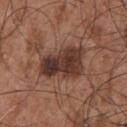The lesion was photographed on a routine skin check and not biopsied; there is no pathology result.
A male subject approximately 55 years of age.
A lesion tile, about 15 mm wide, cut from a 3D total-body photograph.
Located on the chest.
The tile uses white-light illumination.
Approximately 5.5 mm at its widest.